Automated image analysis of the tile measured an outline eccentricity of about 0.8 (0 = round, 1 = elongated) and a symmetry-axis asymmetry near 0.1. And it measured an average lesion color of about L≈45 a*≈24 b*≈31 (CIELAB), about 24 CIELAB-L* units darker than the surrounding skin, and a lesion-to-skin contrast of about 16 (normalized; higher = more distinct). The software also gave a border-irregularity rating of about 1.5/10 and a color-variation rating of about 9.5/10. It also reported a classifier nevus-likeness of about 100/100 and lesion-presence confidence of about 100/100; the lesion is on the upper back; a female subject aged around 35; the recorded lesion diameter is about 5.5 mm; cropped from a total-body skin-imaging series; the visible field is about 15 mm; this is a cross-polarized tile:
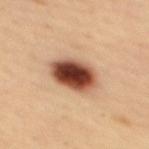<record>
<diagnosis>
  <histopathology>compound melanocytic nevus</histopathology>
  <malignancy>benign</malignancy>
  <taxonomic_path>Benign; Benign melanocytic proliferations; Nevus; Nevus, NOS, Compound</taxonomic_path>
</diagnosis>
</record>